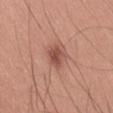biopsy status — no biopsy performed (imaged during a skin exam) | site — the lower back | lesion diameter — ~4 mm (longest diameter) | acquisition — 15 mm crop, total-body photography | patient — male, aged approximately 40 | TBP lesion metrics — an average lesion color of about L≈51 a*≈25 b*≈27 (CIELAB); internal color variation of about 3 on a 0–10 scale and peripheral color asymmetry of about 1; a lesion-detection confidence of about 100/100 | illumination — white-light illumination.